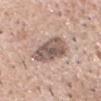Captured during whole-body skin photography for melanoma surveillance; the lesion was not biopsied.
Captured under white-light illumination.
The subject is a male in their 80s.
The lesion is located on the head or neck.
A 15 mm close-up extracted from a 3D total-body photography capture.